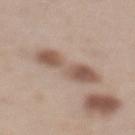<record>
<image>
  <source>total-body photography crop</source>
  <field_of_view_mm>15</field_of_view_mm>
</image>
<site>mid back</site>
<lesion_size>
  <long_diameter_mm_approx>6.5</long_diameter_mm_approx>
</lesion_size>
<patient>
  <sex>female</sex>
  <age_approx>65</age_approx>
</patient>
</record>